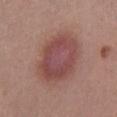* biopsy status · catalogued during a skin exam; not biopsied
* imaging modality · ~15 mm crop, total-body skin-cancer survey
* subject · male, approximately 60 years of age
* body site · the left lower leg